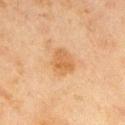A male patient aged around 45.
From the right upper arm.
Automated image analysis of the tile measured a lesion area of about 7.5 mm², a shape eccentricity near 0.65, and a shape-asymmetry score of about 0.2 (0 = symmetric). And it measured an average lesion color of about L≈51 a*≈19 b*≈35 (CIELAB) and a normalized border contrast of about 6.5. It also reported a nevus-likeness score of about 40/100 and lesion-presence confidence of about 100/100.
Cropped from a total-body skin-imaging series; the visible field is about 15 mm.
This is a cross-polarized tile.
The recorded lesion diameter is about 3.5 mm.